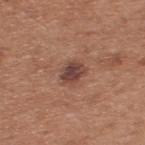Q: Is there a histopathology result?
A: imaged on a skin check; not biopsied
Q: What is the lesion's diameter?
A: ≈3 mm
Q: What lighting was used for the tile?
A: white-light
Q: What are the patient's age and sex?
A: male, about 55 years old
Q: Lesion location?
A: the back
Q: What kind of image is this?
A: ~15 mm crop, total-body skin-cancer survey